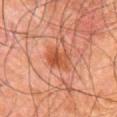follow-up = no biopsy performed (imaged during a skin exam).